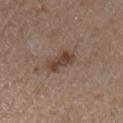Recorded during total-body skin imaging; not selected for excision or biopsy.